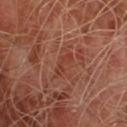The lesion was photographed on a routine skin check and not biopsied; there is no pathology result. Imaged with cross-polarized lighting. About 3 mm across. A 15 mm close-up extracted from a 3D total-body photography capture. A male patient in their mid-60s.